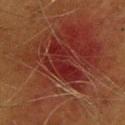Q: Was this lesion biopsied?
A: no biopsy performed (imaged during a skin exam)
Q: Patient demographics?
A: female, about 40 years old
Q: Where on the body is the lesion?
A: the upper back
Q: What is the imaging modality?
A: total-body-photography crop, ~15 mm field of view